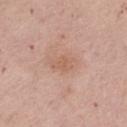Imaged during a routine full-body skin examination; the lesion was not biopsied and no histopathology is available. From the left thigh. This is a white-light tile. Cropped from a total-body skin-imaging series; the visible field is about 15 mm. Longest diameter approximately 3 mm. A female patient aged 63 to 67.